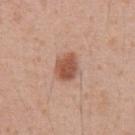<record>
  <biopsy_status>not biopsied; imaged during a skin examination</biopsy_status>
  <patient>
    <sex>male</sex>
    <age_approx>55</age_approx>
  </patient>
  <image>
    <source>total-body photography crop</source>
    <field_of_view_mm>15</field_of_view_mm>
  </image>
  <site>abdomen</site>
</record>